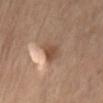follow-up: catalogued during a skin exam; not biopsied
lighting: cross-polarized illumination
body site: the abdomen
image: ~15 mm tile from a whole-body skin photo
patient: male, in their 70s